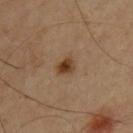The patient is a male in their mid-60s.
The lesion's longest dimension is about 2.5 mm.
The tile uses cross-polarized illumination.
The lesion-visualizer software estimated a border-irregularity rating of about 2.5/10, a within-lesion color-variation index near 4.5/10, and peripheral color asymmetry of about 1.5. The software also gave a detector confidence of about 100 out of 100 that the crop contains a lesion.
A 15 mm close-up tile from a total-body photography series done for melanoma screening.
Located on the upper back.